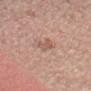Q: Was this lesion biopsied?
A: total-body-photography surveillance lesion; no biopsy
Q: Automated lesion metrics?
A: a shape eccentricity near 0.8 and a shape-asymmetry score of about 0.45 (0 = symmetric); a mean CIELAB color near L≈57 a*≈21 b*≈28, a lesion–skin lightness drop of about 8, and a lesion-to-skin contrast of about 5.5 (normalized; higher = more distinct); a classifier nevus-likeness of about 0/100 and lesion-presence confidence of about 100/100
Q: What are the patient's age and sex?
A: male, approximately 45 years of age
Q: What is the anatomic site?
A: the head or neck
Q: Lesion size?
A: ≈2.5 mm
Q: What lighting was used for the tile?
A: white-light illumination
Q: What kind of image is this?
A: ~15 mm crop, total-body skin-cancer survey